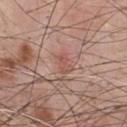No biopsy was performed on this lesion — it was imaged during a full skin examination and was not determined to be concerning.
The tile uses white-light illumination.
A male subject roughly 55 years of age.
From the chest.
The lesion-visualizer software estimated an area of roughly 4 mm², an outline eccentricity of about 0.8 (0 = round, 1 = elongated), and a symmetry-axis asymmetry near 0.25. The software also gave border irregularity of about 2.5 on a 0–10 scale, a within-lesion color-variation index near 2/10, and peripheral color asymmetry of about 0.5. It also reported a nevus-likeness score of about 0/100 and lesion-presence confidence of about 95/100.
A roughly 15 mm field-of-view crop from a total-body skin photograph.
About 2.5 mm across.